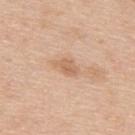Case summary:
– workup: imaged on a skin check; not biopsied
– diameter: about 3 mm
– TBP lesion metrics: radial color variation of about 1
– patient: male, aged 48–52
– image: total-body-photography crop, ~15 mm field of view
– site: the back